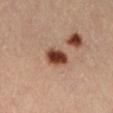Q: Is there a histopathology result?
A: catalogued during a skin exam; not biopsied
Q: Where on the body is the lesion?
A: the right thigh
Q: What did automated image analysis measure?
A: an area of roughly 6.5 mm² and an eccentricity of roughly 0.6; a border-irregularity rating of about 1.5/10 and a peripheral color-asymmetry measure near 1; a classifier nevus-likeness of about 100/100 and a lesion-detection confidence of about 100/100
Q: What is the imaging modality?
A: ~15 mm tile from a whole-body skin photo
Q: Patient demographics?
A: female, in their mid- to late 40s
Q: How was the tile lit?
A: cross-polarized